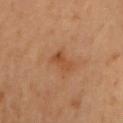  biopsy_status: not biopsied; imaged during a skin examination
  lighting: cross-polarized
  patient:
    sex: male
    age_approx: 55
  automated_metrics:
    area_mm2_approx: 4.5
    shape_asymmetry: 0.4
    cielab_L: 39
    cielab_a: 20
    cielab_b: 31
    vs_skin_darker_L: 6.0
    vs_skin_contrast_norm: 6.0
  site: chest
  lesion_size:
    long_diameter_mm_approx: 3.0
  image:
    source: total-body photography crop
    field_of_view_mm: 15